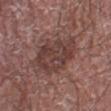<record>
  <biopsy_status>not biopsied; imaged during a skin examination</biopsy_status>
  <lesion_size>
    <long_diameter_mm_approx>6.5</long_diameter_mm_approx>
  </lesion_size>
  <image>
    <source>total-body photography crop</source>
    <field_of_view_mm>15</field_of_view_mm>
  </image>
  <patient>
    <sex>male</sex>
    <age_approx>75</age_approx>
  </patient>
  <site>left lower leg</site>
</record>